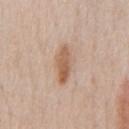Part of a total-body skin-imaging series; this lesion was reviewed on a skin check and was not flagged for biopsy. The lesion's longest dimension is about 5 mm. This is a white-light tile. A male subject approximately 60 years of age. Cropped from a whole-body photographic skin survey; the tile spans about 15 mm. The total-body-photography lesion software estimated an outline eccentricity of about 0.95 (0 = round, 1 = elongated) and a shape-asymmetry score of about 0.2 (0 = symmetric). The analysis additionally found roughly 11 lightness units darker than nearby skin and a normalized border contrast of about 8. The software also gave a border-irregularity index near 2.5/10. The analysis additionally found a classifier nevus-likeness of about 85/100 and a detector confidence of about 100 out of 100 that the crop contains a lesion. The lesion is on the chest.